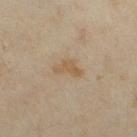This lesion was catalogued during total-body skin photography and was not selected for biopsy.
A female subject, approximately 35 years of age.
A 15 mm close-up tile from a total-body photography series done for melanoma screening.
About 3 mm across.
The tile uses cross-polarized illumination.
An algorithmic analysis of the crop reported a lesion color around L≈54 a*≈13 b*≈32 in CIELAB and a lesion-to-skin contrast of about 6.5 (normalized; higher = more distinct). The software also gave a border-irregularity index near 5/10, a within-lesion color-variation index near 1/10, and radial color variation of about 0.5. The software also gave a classifier nevus-likeness of about 0/100 and a lesion-detection confidence of about 100/100.
On the leg.